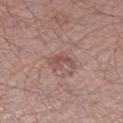Impression:
Captured during whole-body skin photography for melanoma surveillance; the lesion was not biopsied.
Acquisition and patient details:
The total-body-photography lesion software estimated a footprint of about 5 mm². The software also gave an average lesion color of about L≈51 a*≈21 b*≈22 (CIELAB), a lesion–skin lightness drop of about 8, and a normalized border contrast of about 6. A male patient in their mid- to late 50s. The lesion is on the right thigh. The tile uses white-light illumination. Longest diameter approximately 3.5 mm. Cropped from a total-body skin-imaging series; the visible field is about 15 mm.